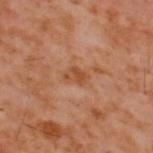| field | value |
|---|---|
| biopsy status | catalogued during a skin exam; not biopsied |
| image | ~15 mm crop, total-body skin-cancer survey |
| illumination | cross-polarized |
| lesion size | about 2.5 mm |
| location | the upper back |
| automated metrics | a footprint of about 4 mm², a shape eccentricity near 0.75, and a symmetry-axis asymmetry near 0.35; a lesion color around L≈45 a*≈23 b*≈34 in CIELAB, roughly 7 lightness units darker than nearby skin, and a lesion-to-skin contrast of about 7 (normalized; higher = more distinct) |
| subject | male, aged 58–62 |